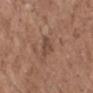Findings:
* workup · imaged on a skin check; not biopsied
* patient · female, in their mid- to late 60s
* image · total-body-photography crop, ~15 mm field of view
* anatomic site · the arm
* diameter · ≈2.5 mm
* illumination · white-light